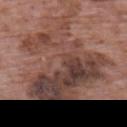biopsy_status: not biopsied; imaged during a skin examination
lighting: white-light
patient:
  sex: male
  age_approx: 70
lesion_size:
  long_diameter_mm_approx: 11.0
image:
  source: total-body photography crop
  field_of_view_mm: 15
site: upper back